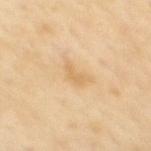Clinical impression: The lesion was photographed on a routine skin check and not biopsied; there is no pathology result. Context: Located on the upper back. The subject is a female approximately 45 years of age. A roughly 15 mm field-of-view crop from a total-body skin photograph.